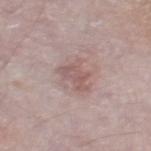The lesion is on the right thigh.
A male subject, approximately 75 years of age.
The tile uses white-light illumination.
A 15 mm crop from a total-body photograph taken for skin-cancer surveillance.
An algorithmic analysis of the crop reported a shape-asymmetry score of about 0.45 (0 = symmetric). The analysis additionally found border irregularity of about 5 on a 0–10 scale.